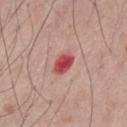Impression:
Imaged during a routine full-body skin examination; the lesion was not biopsied and no histopathology is available.
Clinical summary:
The lesion is located on the mid back. A 15 mm crop from a total-body photograph taken for skin-cancer surveillance. The lesion-visualizer software estimated a border-irregularity rating of about 1.5/10, a color-variation rating of about 4.5/10, and radial color variation of about 1.5. The lesion's longest dimension is about 2.5 mm. A male subject, aged 33–37. This is a white-light tile.